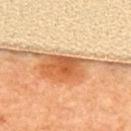notes: no biopsy performed (imaged during a skin exam)
size: about 3 mm
acquisition: total-body-photography crop, ~15 mm field of view
subject: female, aged 63 to 67
body site: the back